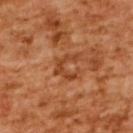Captured during whole-body skin photography for melanoma surveillance; the lesion was not biopsied.
An algorithmic analysis of the crop reported an area of roughly 5 mm² and two-axis asymmetry of about 0.7. The analysis additionally found a mean CIELAB color near L≈46 a*≈29 b*≈40, roughly 9 lightness units darker than nearby skin, and a normalized border contrast of about 7. It also reported a classifier nevus-likeness of about 0/100 and lesion-presence confidence of about 100/100.
A 15 mm close-up extracted from a 3D total-body photography capture.
From the upper back.
The lesion's longest dimension is about 3 mm.
The patient is a female approximately 55 years of age.